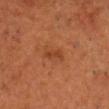Imaged during a routine full-body skin examination; the lesion was not biopsied and no histopathology is available.
Automated tile analysis of the lesion measured an average lesion color of about L≈34 a*≈23 b*≈32 (CIELAB), about 6 CIELAB-L* units darker than the surrounding skin, and a normalized border contrast of about 6.
Captured under cross-polarized illumination.
The lesion is on the head or neck.
The recorded lesion diameter is about 2.5 mm.
A 15 mm close-up extracted from a 3D total-body photography capture.
A male patient, roughly 65 years of age.